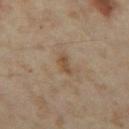Captured during whole-body skin photography for melanoma surveillance; the lesion was not biopsied.
A 15 mm crop from a total-body photograph taken for skin-cancer surveillance.
This is a cross-polarized tile.
From the left thigh.
A female patient, in their mid-30s.
The lesion's longest dimension is about 2.5 mm.